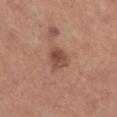<tbp_lesion>
<biopsy_status>not biopsied; imaged during a skin examination</biopsy_status>
<image>
  <source>total-body photography crop</source>
  <field_of_view_mm>15</field_of_view_mm>
</image>
<site>left lower leg</site>
<patient>
  <sex>female</sex>
  <age_approx>45</age_approx>
</patient>
<automated_metrics>
  <area_mm2_approx>6.5</area_mm2_approx>
  <shape_asymmetry>0.25</shape_asymmetry>
  <border_irregularity_0_10>2.0</border_irregularity_0_10>
  <color_variation_0_10>3.5</color_variation_0_10>
  <peripheral_color_asymmetry>1.0</peripheral_color_asymmetry>
  <nevus_likeness_0_100>65</nevus_likeness_0_100>
  <lesion_detection_confidence_0_100>100</lesion_detection_confidence_0_100>
</automated_metrics>
<lighting>white-light</lighting>
<lesion_size>
  <long_diameter_mm_approx>3.0</long_diameter_mm_approx>
</lesion_size>
</tbp_lesion>